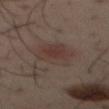Impression: This lesion was catalogued during total-body skin photography and was not selected for biopsy. Clinical summary: The tile uses cross-polarized illumination. Cropped from a whole-body photographic skin survey; the tile spans about 15 mm. Located on the front of the torso. A male patient roughly 40 years of age. An algorithmic analysis of the crop reported an area of roughly 13 mm², a shape eccentricity near 0.7, and a symmetry-axis asymmetry near 0.15. The software also gave a mean CIELAB color near L≈35 a*≈15 b*≈20 and roughly 6 lightness units darker than nearby skin. The software also gave a nevus-likeness score of about 80/100 and lesion-presence confidence of about 100/100. Longest diameter approximately 5 mm.